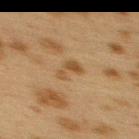No biopsy was performed on this lesion — it was imaged during a full skin examination and was not determined to be concerning. Automated image analysis of the tile measured a mean CIELAB color near L≈43 a*≈14 b*≈32 and about 6 CIELAB-L* units darker than the surrounding skin. The software also gave internal color variation of about 4.5 on a 0–10 scale. The recorded lesion diameter is about 4 mm. A female subject aged approximately 40. The lesion is on the upper back. A 15 mm crop from a total-body photograph taken for skin-cancer surveillance.